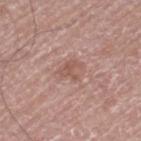Part of a total-body skin-imaging series; this lesion was reviewed on a skin check and was not flagged for biopsy. The tile uses white-light illumination. About 3 mm across. A male patient, aged 73–77. A roughly 15 mm field-of-view crop from a total-body skin photograph. On the left thigh.